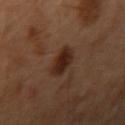No biopsy was performed on this lesion — it was imaged during a full skin examination and was not determined to be concerning. Longest diameter approximately 3.5 mm. A male subject, about 50 years old. Automated image analysis of the tile measured an area of roughly 7.5 mm², an eccentricity of roughly 0.65, and a shape-asymmetry score of about 0.15 (0 = symmetric). The software also gave an average lesion color of about L≈25 a*≈18 b*≈24 (CIELAB), a lesion–skin lightness drop of about 10, and a normalized border contrast of about 10.5. The analysis additionally found a border-irregularity rating of about 1.5/10, a within-lesion color-variation index near 3.5/10, and radial color variation of about 1. The tile uses cross-polarized illumination. A region of skin cropped from a whole-body photographic capture, roughly 15 mm wide. On the left arm.